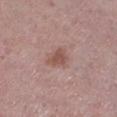No biopsy was performed on this lesion — it was imaged during a full skin examination and was not determined to be concerning. Imaged with white-light lighting. Cropped from a whole-body photographic skin survey; the tile spans about 15 mm. The subject is a male about 75 years old. From the left lower leg.